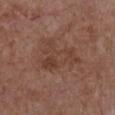Clinical impression: The lesion was tiled from a total-body skin photograph and was not biopsied. Background: Approximately 5.5 mm at its widest. This is a white-light tile. On the chest. A female subject roughly 80 years of age. A 15 mm close-up extracted from a 3D total-body photography capture.